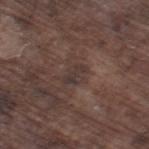The lesion was tiled from a total-body skin photograph and was not biopsied.
This is a white-light tile.
From the right thigh.
The recorded lesion diameter is about 2.5 mm.
A male subject, aged 73 to 77.
A 15 mm crop from a total-body photograph taken for skin-cancer surveillance.